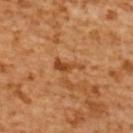follow-up = no biopsy performed (imaged during a skin exam); acquisition = ~15 mm crop, total-body skin-cancer survey; patient = female, aged approximately 55; body site = the upper back.